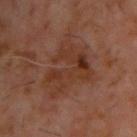notes: catalogued during a skin exam; not biopsied | illumination: cross-polarized illumination | subject: male, in their 60s | diameter: ~7 mm (longest diameter) | site: the upper back | acquisition: total-body-photography crop, ~15 mm field of view.